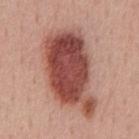Clinical impression: No biopsy was performed on this lesion — it was imaged during a full skin examination and was not determined to be concerning. Clinical summary: The lesion is on the mid back. Automated image analysis of the tile measured an average lesion color of about L≈48 a*≈26 b*≈26 (CIELAB), about 18 CIELAB-L* units darker than the surrounding skin, and a normalized lesion–skin contrast near 12. And it measured a border-irregularity rating of about 3/10 and a color-variation rating of about 6.5/10. And it measured a lesion-detection confidence of about 100/100. The subject is a male in their mid-50s. A lesion tile, about 15 mm wide, cut from a 3D total-body photograph.